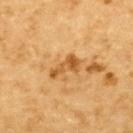Clinical impression: The lesion was photographed on a routine skin check and not biopsied; there is no pathology result. Clinical summary: The lesion is located on the upper back. Cropped from a whole-body photographic skin survey; the tile spans about 15 mm. Longest diameter approximately 3.5 mm. A male patient aged around 85 or older.